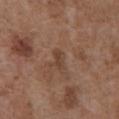Notes:
• workup · no biopsy performed (imaged during a skin exam)
• image · 15 mm crop, total-body photography
• subject · male, aged 73–77
• site · the abdomen
• tile lighting · white-light illumination
• size · about 2.5 mm